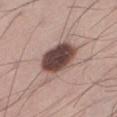| key | value |
|---|---|
| notes | catalogued during a skin exam; not biopsied |
| location | the left lower leg |
| image source | total-body-photography crop, ~15 mm field of view |
| subject | male, aged 33–37 |
| illumination | white-light illumination |
| automated lesion analysis | a mean CIELAB color near L≈45 a*≈18 b*≈20 and about 20 CIELAB-L* units darker than the surrounding skin; radial color variation of about 1.5 |
| size | about 5.5 mm |